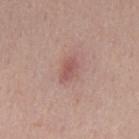| feature | finding |
|---|---|
| workup | catalogued during a skin exam; not biopsied |